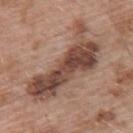{
  "biopsy_status": "not biopsied; imaged during a skin examination",
  "lesion_size": {
    "long_diameter_mm_approx": 10.5
  },
  "lighting": "white-light",
  "patient": {
    "sex": "male",
    "age_approx": 55
  },
  "site": "back",
  "image": {
    "source": "total-body photography crop",
    "field_of_view_mm": 15
  }
}